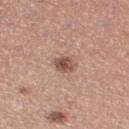biopsy_status: not biopsied; imaged during a skin examination
automated_metrics:
  area_mm2_approx: 4.5
  eccentricity: 0.55
  shape_asymmetry: 0.25
  cielab_L: 52
  cielab_a: 20
  cielab_b: 25
  vs_skin_contrast_norm: 8.5
  border_irregularity_0_10: 2.0
  color_variation_0_10: 5.0
  peripheral_color_asymmetry: 1.5
lighting: white-light
image:
  source: total-body photography crop
  field_of_view_mm: 15
site: right thigh
patient:
  sex: female
  age_approx: 30
lesion_size:
  long_diameter_mm_approx: 2.5